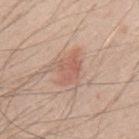notes: catalogued during a skin exam; not biopsied.